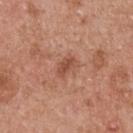Assessment: Part of a total-body skin-imaging series; this lesion was reviewed on a skin check and was not flagged for biopsy. Context: A male subject aged 53 to 57. A lesion tile, about 15 mm wide, cut from a 3D total-body photograph. From the upper back.